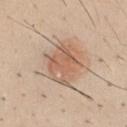Recorded during total-body skin imaging; not selected for excision or biopsy.
A region of skin cropped from a whole-body photographic capture, roughly 15 mm wide.
Located on the chest.
The subject is a male about 35 years old.